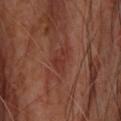<tbp_lesion>
<biopsy_status>not biopsied; imaged during a skin examination</biopsy_status>
<patient>
  <sex>male</sex>
  <age_approx>65</age_approx>
</patient>
<lighting>cross-polarized</lighting>
<image>
  <source>total-body photography crop</source>
  <field_of_view_mm>15</field_of_view_mm>
</image>
<site>upper back</site>
<lesion_size>
  <long_diameter_mm_approx>3.5</long_diameter_mm_approx>
</lesion_size>
</tbp_lesion>